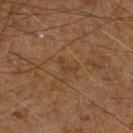Q: Was this lesion biopsied?
A: catalogued during a skin exam; not biopsied
Q: What is the imaging modality?
A: ~15 mm crop, total-body skin-cancer survey
Q: How was the tile lit?
A: cross-polarized illumination
Q: What is the anatomic site?
A: the leg
Q: What are the patient's age and sex?
A: male, aged 58 to 62